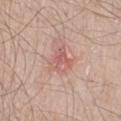Clinical summary:
The lesion is located on the right lower leg. Cropped from a whole-body photographic skin survey; the tile spans about 15 mm. A male subject, aged approximately 60. Longest diameter approximately 3 mm. Automated tile analysis of the lesion measured about 8 CIELAB-L* units darker than the surrounding skin and a normalized border contrast of about 5. It also reported border irregularity of about 3.5 on a 0–10 scale, a within-lesion color-variation index near 4/10, and peripheral color asymmetry of about 1.5.